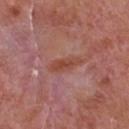follow-up: imaged on a skin check; not biopsied